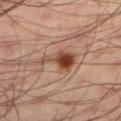biopsy status — imaged on a skin check; not biopsied
body site — the left lower leg
imaging modality — ~15 mm tile from a whole-body skin photo
lesion diameter — ≈5 mm
lighting — cross-polarized
subject — male, in their mid-30s